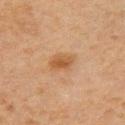Recorded during total-body skin imaging; not selected for excision or biopsy. An algorithmic analysis of the crop reported a mean CIELAB color near L≈45 a*≈18 b*≈33, a lesion–skin lightness drop of about 7, and a lesion-to-skin contrast of about 7 (normalized; higher = more distinct). The analysis additionally found a border-irregularity rating of about 2/10, internal color variation of about 4 on a 0–10 scale, and peripheral color asymmetry of about 1. And it measured a classifier nevus-likeness of about 85/100 and a lesion-detection confidence of about 100/100. On the right upper arm. Longest diameter approximately 3 mm. A male subject roughly 70 years of age. This image is a 15 mm lesion crop taken from a total-body photograph. Imaged with cross-polarized lighting.